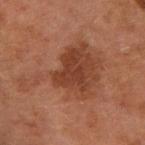The lesion was tiled from a total-body skin photograph and was not biopsied.
A 15 mm crop from a total-body photograph taken for skin-cancer surveillance.
From the left forearm.
Measured at roughly 9.5 mm in maximum diameter.
A female patient, roughly 60 years of age.